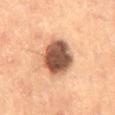Image and clinical context:
A 15 mm close-up tile from a total-body photography series done for melanoma screening. A male subject, aged around 60. This is a cross-polarized tile. The total-body-photography lesion software estimated an area of roughly 17 mm², an eccentricity of roughly 0.7, and two-axis asymmetry of about 0.1. And it measured a within-lesion color-variation index near 6.5/10. About 5.5 mm across.